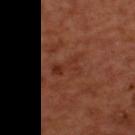TBP lesion metrics — a mean CIELAB color near L≈33 a*≈25 b*≈29 and a lesion-to-skin contrast of about 5 (normalized; higher = more distinct); a nevus-likeness score of about 0/100 and a lesion-detection confidence of about 100/100
size — ~4.5 mm (longest diameter)
anatomic site — the upper back
imaging modality — total-body-photography crop, ~15 mm field of view
subject — male, about 50 years old
lighting — cross-polarized